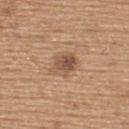notes: total-body-photography surveillance lesion; no biopsy
image source: ~15 mm tile from a whole-body skin photo
lesion size: ≈3.5 mm
site: the upper back
patient: male, about 65 years old
automated metrics: an eccentricity of roughly 0.55 and a symmetry-axis asymmetry near 0.2; about 10 CIELAB-L* units darker than the surrounding skin and a normalized lesion–skin contrast near 7